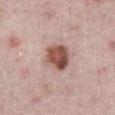Q: Was a biopsy performed?
A: no biopsy performed (imaged during a skin exam)
Q: What did automated image analysis measure?
A: a footprint of about 9.5 mm², a shape eccentricity near 0.5, and a shape-asymmetry score of about 0.15 (0 = symmetric); an average lesion color of about L≈52 a*≈22 b*≈25 (CIELAB), about 16 CIELAB-L* units darker than the surrounding skin, and a normalized border contrast of about 11
Q: What lighting was used for the tile?
A: white-light illumination
Q: Patient demographics?
A: male, in their mid-70s
Q: Lesion location?
A: the front of the torso
Q: What kind of image is this?
A: ~15 mm tile from a whole-body skin photo